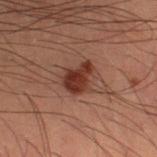Clinical impression: Captured during whole-body skin photography for melanoma surveillance; the lesion was not biopsied. Image and clinical context: A male patient in their mid- to late 50s. Cropped from a whole-body photographic skin survey; the tile spans about 15 mm. This is a cross-polarized tile. From the left thigh. Approximately 4 mm at its widest. The total-body-photography lesion software estimated internal color variation of about 3 on a 0–10 scale and radial color variation of about 1.